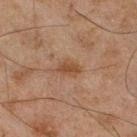Part of a total-body skin-imaging series; this lesion was reviewed on a skin check and was not flagged for biopsy. Measured at roughly 3 mm in maximum diameter. A lesion tile, about 15 mm wide, cut from a 3D total-body photograph. This is a cross-polarized tile. A male subject approximately 45 years of age. The lesion is located on the right lower leg. Automated tile analysis of the lesion measured a lesion area of about 4 mm² and two-axis asymmetry of about 0.3.